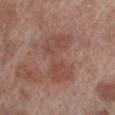biopsy_status: not biopsied; imaged during a skin examination
lighting: white-light
lesion_size:
  long_diameter_mm_approx: 6.0
image:
  source: total-body photography crop
  field_of_view_mm: 15
automated_metrics:
  cielab_L: 47
  cielab_a: 23
  cielab_b: 25
  color_variation_0_10: 3.0
  peripheral_color_asymmetry: 1.0
  nevus_likeness_0_100: 0
site: left lower leg
patient:
  sex: male
  age_approx: 70